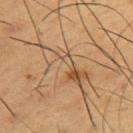workup: total-body-photography surveillance lesion; no biopsy
lesion size: ≈7.5 mm
image-analysis metrics: a footprint of about 11 mm²
imaging modality: ~15 mm tile from a whole-body skin photo
anatomic site: the right upper arm
patient: male, in their mid-50s
lighting: cross-polarized